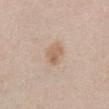Findings:
* biopsy status: no biopsy performed (imaged during a skin exam)
* automated lesion analysis: a mean CIELAB color near L≈62 a*≈16 b*≈30, a lesion–skin lightness drop of about 8, and a normalized border contrast of about 6.5; a color-variation rating of about 3.5/10
* subject: male, about 50 years old
* acquisition: ~15 mm tile from a whole-body skin photo
* anatomic site: the abdomen